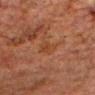| feature | finding |
|---|---|
| workup | catalogued during a skin exam; not biopsied |
| size | about 3 mm |
| image | ~15 mm crop, total-body skin-cancer survey |
| site | the head or neck |
| illumination | cross-polarized illumination |
| patient | male, roughly 60 years of age |